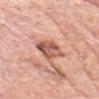- image · ~15 mm tile from a whole-body skin photo
- lighting · white-light
- diameter · about 4 mm
- site · the head or neck
- subject · male, approximately 75 years of age